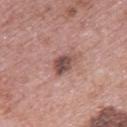Impression: This lesion was catalogued during total-body skin photography and was not selected for biopsy. Image and clinical context: The recorded lesion diameter is about 3 mm. From the upper back. This image is a 15 mm lesion crop taken from a total-body photograph. Captured under white-light illumination. A female patient about 60 years old.